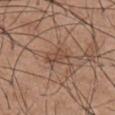Automated image analysis of the tile measured a mean CIELAB color near L≈48 a*≈18 b*≈28 and a normalized border contrast of about 6. The lesion's longest dimension is about 3 mm. This image is a 15 mm lesion crop taken from a total-body photograph. Captured under white-light illumination. From the front of the torso. A male subject, aged around 55.